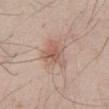| key | value |
|---|---|
| follow-up | no biopsy performed (imaged during a skin exam) |
| subject | male, roughly 50 years of age |
| imaging modality | ~15 mm crop, total-body skin-cancer survey |
| body site | the abdomen |